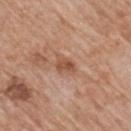Recorded during total-body skin imaging; not selected for excision or biopsy.